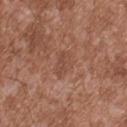Recorded during total-body skin imaging; not selected for excision or biopsy. The lesion-visualizer software estimated a mean CIELAB color near L≈48 a*≈22 b*≈28 and a lesion-to-skin contrast of about 5 (normalized; higher = more distinct). And it measured a border-irregularity rating of about 2.5/10, a within-lesion color-variation index near 2/10, and radial color variation of about 1. Imaged with white-light lighting. A 15 mm close-up extracted from a 3D total-body photography capture. The subject is a male aged around 45. From the upper back. Longest diameter approximately 3 mm.